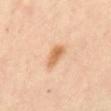Q: Was a biopsy performed?
A: catalogued during a skin exam; not biopsied
Q: What kind of image is this?
A: ~15 mm tile from a whole-body skin photo
Q: What are the patient's age and sex?
A: female, in their 60s
Q: Where on the body is the lesion?
A: the abdomen